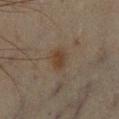Findings:
– notes: catalogued during a skin exam; not biopsied
– body site: the right lower leg
– diameter: ~2.5 mm (longest diameter)
– lighting: cross-polarized
– subject: male, approximately 45 years of age
– image-analysis metrics: a mean CIELAB color near L≈31 a*≈12 b*≈23 and a normalized border contrast of about 7.5; peripheral color asymmetry of about 0.5
– image: ~15 mm tile from a whole-body skin photo